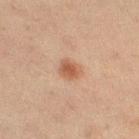Assessment:
Captured during whole-body skin photography for melanoma surveillance; the lesion was not biopsied.
Acquisition and patient details:
The lesion's longest dimension is about 2.5 mm. The subject is a female aged 18–22. An algorithmic analysis of the crop reported a footprint of about 5 mm², a shape eccentricity near 0.5, and a shape-asymmetry score of about 0.2 (0 = symmetric). The software also gave an average lesion color of about L≈49 a*≈20 b*≈29 (CIELAB), roughly 9 lightness units darker than nearby skin, and a lesion-to-skin contrast of about 7.5 (normalized; higher = more distinct). The software also gave a border-irregularity rating of about 2/10 and a peripheral color-asymmetry measure near 0.5. The software also gave a nevus-likeness score of about 90/100 and lesion-presence confidence of about 100/100. From the left thigh. The tile uses cross-polarized illumination. A region of skin cropped from a whole-body photographic capture, roughly 15 mm wide.